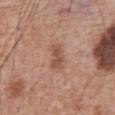Findings:
* notes — catalogued during a skin exam; not biopsied
* subject — male, approximately 60 years of age
* location — the front of the torso
* automated lesion analysis — a classifier nevus-likeness of about 10/100 and a detector confidence of about 100 out of 100 that the crop contains a lesion
* lesion size — ≈3.5 mm
* image source — 15 mm crop, total-body photography